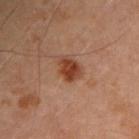No biopsy was performed on this lesion — it was imaged during a full skin examination and was not determined to be concerning.
The patient is a male roughly 50 years of age.
Located on the left upper arm.
A region of skin cropped from a whole-body photographic capture, roughly 15 mm wide.
Imaged with cross-polarized lighting.
The recorded lesion diameter is about 3 mm.